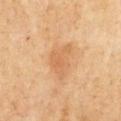Part of a total-body skin-imaging series; this lesion was reviewed on a skin check and was not flagged for biopsy. A 15 mm crop from a total-body photograph taken for skin-cancer surveillance. The total-body-photography lesion software estimated a mean CIELAB color near L≈60 a*≈21 b*≈38, a lesion–skin lightness drop of about 7, and a normalized border contrast of about 5. The analysis additionally found a border-irregularity index near 4/10 and radial color variation of about 1. The subject is a male aged 68 to 72. Captured under cross-polarized illumination. The lesion is located on the chest. Measured at roughly 4.5 mm in maximum diameter.